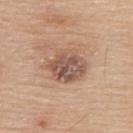{
  "biopsy_status": "not biopsied; imaged during a skin examination",
  "patient": {
    "sex": "male",
    "age_approx": 80
  },
  "lesion_size": {
    "long_diameter_mm_approx": 5.0
  },
  "site": "upper back",
  "lighting": "white-light",
  "image": {
    "source": "total-body photography crop",
    "field_of_view_mm": 15
  },
  "automated_metrics": {
    "border_irregularity_0_10": 2.5,
    "color_variation_0_10": 6.5,
    "peripheral_color_asymmetry": 2.0
  }
}